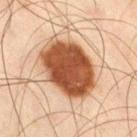The lesion was tiled from a total-body skin photograph and was not biopsied.
A 15 mm crop from a total-body photograph taken for skin-cancer surveillance.
The lesion is located on the right thigh.
About 7.5 mm across.
The patient is a male in their mid- to late 40s.